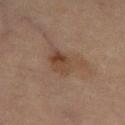Captured during whole-body skin photography for melanoma surveillance; the lesion was not biopsied. Located on the left thigh. The subject is a female aged approximately 70. Approximately 4.5 mm at its widest. A 15 mm close-up extracted from a 3D total-body photography capture. Automated image analysis of the tile measured a mean CIELAB color near L≈37 a*≈15 b*≈25 and a normalized border contrast of about 6.5. It also reported a border-irregularity index near 5/10 and a color-variation rating of about 5.5/10. The analysis additionally found a nevus-likeness score of about 5/100 and a lesion-detection confidence of about 100/100.